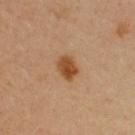The lesion was tiled from a total-body skin photograph and was not biopsied. Captured under cross-polarized illumination. The subject is a female aged 48 to 52. A 15 mm close-up extracted from a 3D total-body photography capture.